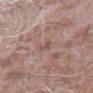{
  "biopsy_status": "not biopsied; imaged during a skin examination"
}